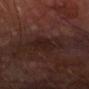Impression:
The lesion was photographed on a routine skin check and not biopsied; there is no pathology result.
Clinical summary:
About 3 mm across. A 15 mm close-up tile from a total-body photography series done for melanoma screening. Automated image analysis of the tile measured an area of roughly 6 mm², an eccentricity of roughly 0.65, and two-axis asymmetry of about 0.2. And it measured a lesion color around L≈18 a*≈17 b*≈17 in CIELAB, about 5 CIELAB-L* units darker than the surrounding skin, and a lesion-to-skin contrast of about 6 (normalized; higher = more distinct). The analysis additionally found a nevus-likeness score of about 5/100 and a lesion-detection confidence of about 100/100. A male patient about 70 years old. Located on the right forearm. This is a cross-polarized tile.